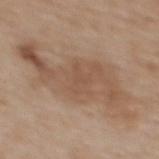Notes:
- notes: total-body-photography surveillance lesion; no biopsy
- diameter: about 12.5 mm
- subject: female, aged 63 to 67
- image: total-body-photography crop, ~15 mm field of view
- location: the back